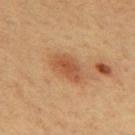biopsy status: imaged on a skin check; not biopsied | diameter: about 4 mm | tile lighting: cross-polarized | subject: male, aged 58–62 | location: the mid back | image: 15 mm crop, total-body photography.